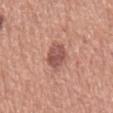follow-up = imaged on a skin check; not biopsied | site = the mid back | acquisition = ~15 mm crop, total-body skin-cancer survey | lighting = white-light | diameter = ~4 mm (longest diameter) | patient = male, in their mid- to late 70s | image-analysis metrics = an area of roughly 8 mm², a shape eccentricity near 0.75, and a symmetry-axis asymmetry near 0.15; a lesion color around L≈53 a*≈24 b*≈26 in CIELAB; a classifier nevus-likeness of about 65/100.